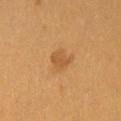No biopsy was performed on this lesion — it was imaged during a full skin examination and was not determined to be concerning. An algorithmic analysis of the crop reported a border-irregularity index near 2.5/10. A female subject aged 48 to 52. This is a cross-polarized tile. This image is a 15 mm lesion crop taken from a total-body photograph. From the chest. The lesion's longest dimension is about 3 mm.